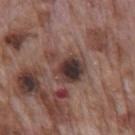<record>
  <biopsy_status>not biopsied; imaged during a skin examination</biopsy_status>
  <patient>
    <sex>male</sex>
    <age_approx>70</age_approx>
  </patient>
  <site>back</site>
  <automated_metrics>
    <color_variation_0_10>9.0</color_variation_0_10>
    <peripheral_color_asymmetry>2.5</peripheral_color_asymmetry>
  </automated_metrics>
  <image>
    <source>total-body photography crop</source>
    <field_of_view_mm>15</field_of_view_mm>
  </image>
  <lighting>white-light</lighting>
</record>